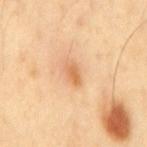Clinical impression:
Part of a total-body skin-imaging series; this lesion was reviewed on a skin check and was not flagged for biopsy.
Acquisition and patient details:
A male subject aged 38–42. Imaged with cross-polarized lighting. A close-up tile cropped from a whole-body skin photograph, about 15 mm across. From the back. Measured at roughly 2.5 mm in maximum diameter.